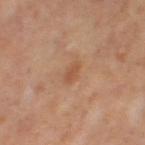{
  "biopsy_status": "not biopsied; imaged during a skin examination",
  "site": "left thigh",
  "patient": {
    "sex": "female",
    "age_approx": 50
  },
  "image": {
    "source": "total-body photography crop",
    "field_of_view_mm": 15
  },
  "automated_metrics": {
    "eccentricity": 0.8,
    "shape_asymmetry": 0.3,
    "border_irregularity_0_10": 2.5,
    "color_variation_0_10": 1.5,
    "peripheral_color_asymmetry": 0.5,
    "lesion_detection_confidence_0_100": 100
  },
  "lesion_size": {
    "long_diameter_mm_approx": 2.5
  },
  "lighting": "cross-polarized"
}